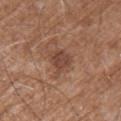The tile uses white-light illumination. The total-body-photography lesion software estimated a mean CIELAB color near L≈44 a*≈21 b*≈27, about 9 CIELAB-L* units darker than the surrounding skin, and a lesion-to-skin contrast of about 7.5 (normalized; higher = more distinct). It also reported a detector confidence of about 100 out of 100 that the crop contains a lesion. A close-up tile cropped from a whole-body skin photograph, about 15 mm across. The patient is a male approximately 60 years of age. Approximately 3 mm at its widest. Located on the left upper arm.